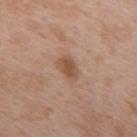notes=total-body-photography surveillance lesion; no biopsy | tile lighting=white-light illumination | body site=the mid back | imaging modality=15 mm crop, total-body photography | patient=male, in their 50s.